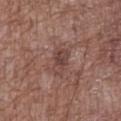biopsy status: catalogued during a skin exam; not biopsied
location: the abdomen
lighting: white-light
patient: male, approximately 70 years of age
acquisition: 15 mm crop, total-body photography